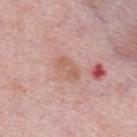  site: upper back
  lighting: white-light
  image:
    source: total-body photography crop
    field_of_view_mm: 15
  patient:
    sex: female
    age_approx: 65
  automated_metrics:
    area_mm2_approx: 4.0
    eccentricity: 0.9
    shape_asymmetry: 0.3
    border_irregularity_0_10: 3.5